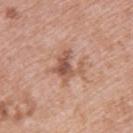Q: Is there a histopathology result?
A: no biopsy performed (imaged during a skin exam)
Q: How large is the lesion?
A: about 3.5 mm
Q: Who is the patient?
A: female, aged 38 to 42
Q: Lesion location?
A: the upper back
Q: Illumination type?
A: white-light
Q: How was this image acquired?
A: ~15 mm tile from a whole-body skin photo